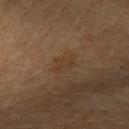{"biopsy_status": "not biopsied; imaged during a skin examination", "lesion_size": {"long_diameter_mm_approx": 3.0}, "automated_metrics": {"area_mm2_approx": 4.0, "eccentricity": 0.75, "shape_asymmetry": 0.35}, "site": "left forearm", "lighting": "cross-polarized", "image": {"source": "total-body photography crop", "field_of_view_mm": 15}, "patient": {"sex": "female", "age_approx": 50}}